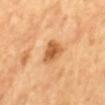biopsy status: catalogued during a skin exam; not biopsied
patient: male, about 65 years old
location: the back
imaging modality: ~15 mm tile from a whole-body skin photo
diameter: about 3.5 mm